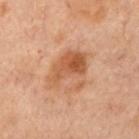Part of a total-body skin-imaging series; this lesion was reviewed on a skin check and was not flagged for biopsy.
A female patient, aged 53–57.
A region of skin cropped from a whole-body photographic capture, roughly 15 mm wide.
Located on the chest.
The tile uses cross-polarized illumination.